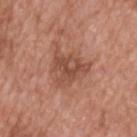<case>
<biopsy_status>not biopsied; imaged during a skin examination</biopsy_status>
<lighting>white-light</lighting>
<lesion_size>
  <long_diameter_mm_approx>4.5</long_diameter_mm_approx>
</lesion_size>
<image>
  <source>total-body photography crop</source>
  <field_of_view_mm>15</field_of_view_mm>
</image>
<automated_metrics>
  <eccentricity>0.65</eccentricity>
  <shape_asymmetry>0.4</shape_asymmetry>
  <nevus_likeness_0_100>10</nevus_likeness_0_100>
  <lesion_detection_confidence_0_100>100</lesion_detection_confidence_0_100>
</automated_metrics>
<site>upper back</site>
<patient>
  <sex>male</sex>
  <age_approx>50</age_approx>
</patient>
</case>